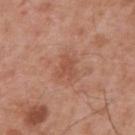No biopsy was performed on this lesion — it was imaged during a full skin examination and was not determined to be concerning. A male patient aged around 55. The lesion is on the upper back. The recorded lesion diameter is about 2.5 mm. A region of skin cropped from a whole-body photographic capture, roughly 15 mm wide. The total-body-photography lesion software estimated an area of roughly 4.5 mm², an eccentricity of roughly 0.5, and a shape-asymmetry score of about 0.35 (0 = symmetric). And it measured a mean CIELAB color near L≈51 a*≈25 b*≈31, roughly 7 lightness units darker than nearby skin, and a lesion-to-skin contrast of about 5.5 (normalized; higher = more distinct). It also reported a border-irregularity index near 4/10, internal color variation of about 1 on a 0–10 scale, and radial color variation of about 0.5.